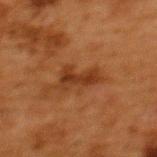Captured during whole-body skin photography for melanoma surveillance; the lesion was not biopsied. The lesion's longest dimension is about 4.5 mm. From the upper back. This is a cross-polarized tile. The patient is a female about 50 years old. This image is a 15 mm lesion crop taken from a total-body photograph.